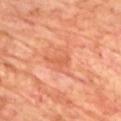Assessment: The lesion was photographed on a routine skin check and not biopsied; there is no pathology result. Image and clinical context: The subject is a male about 70 years old. From the chest. The total-body-photography lesion software estimated a footprint of about 2.5 mm² and two-axis asymmetry of about 0.4. And it measured a border-irregularity index near 4/10, a color-variation rating of about 0/10, and a peripheral color-asymmetry measure near 0. The software also gave an automated nevus-likeness rating near 0 out of 100 and a detector confidence of about 100 out of 100 that the crop contains a lesion. The tile uses cross-polarized illumination. The lesion's longest dimension is about 2.5 mm. A lesion tile, about 15 mm wide, cut from a 3D total-body photograph.